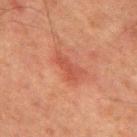<case>
  <biopsy_status>not biopsied; imaged during a skin examination</biopsy_status>
  <patient>
    <sex>male</sex>
    <age_approx>65</age_approx>
  </patient>
  <image>
    <source>total-body photography crop</source>
    <field_of_view_mm>15</field_of_view_mm>
  </image>
  <site>chest</site>
  <automated_metrics>
    <area_mm2_approx>6.0</area_mm2_approx>
    <eccentricity>0.9</eccentricity>
    <cielab_L>42</cielab_L>
    <cielab_a>26</cielab_a>
    <cielab_b>28</cielab_b>
    <vs_skin_darker_L>7.0</vs_skin_darker_L>
    <vs_skin_contrast_norm>5.5</vs_skin_contrast_norm>
    <nevus_likeness_0_100>0</nevus_likeness_0_100>
    <lesion_detection_confidence_0_100>100</lesion_detection_confidence_0_100>
  </automated_metrics>
</case>